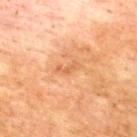Assessment:
Recorded during total-body skin imaging; not selected for excision or biopsy.
Image and clinical context:
An algorithmic analysis of the crop reported a lesion area of about 3 mm², a shape eccentricity near 0.9, and two-axis asymmetry of about 0.45. Captured under cross-polarized illumination. A male patient aged approximately 75. Approximately 3 mm at its widest. A region of skin cropped from a whole-body photographic capture, roughly 15 mm wide. Located on the upper back.